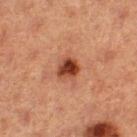  biopsy_status: not biopsied; imaged during a skin examination
  lesion_size:
    long_diameter_mm_approx: 3.0
  site: leg
  patient:
    sex: female
    age_approx: 40
  lighting: cross-polarized
  image:
    source: total-body photography crop
    field_of_view_mm: 15